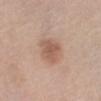Assessment:
Captured during whole-body skin photography for melanoma surveillance; the lesion was not biopsied.
Acquisition and patient details:
Longest diameter approximately 3.5 mm. From the abdomen. A female subject aged 58–62. Imaged with white-light lighting. A close-up tile cropped from a whole-body skin photograph, about 15 mm across.